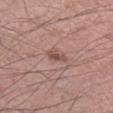Q: Is there a histopathology result?
A: imaged on a skin check; not biopsied
Q: What is the lesion's diameter?
A: about 3 mm
Q: Patient demographics?
A: male, roughly 40 years of age
Q: What is the anatomic site?
A: the right lower leg
Q: What kind of image is this?
A: ~15 mm crop, total-body skin-cancer survey
Q: How was the tile lit?
A: white-light
Q: Automated lesion metrics?
A: about 10 CIELAB-L* units darker than the surrounding skin and a normalized lesion–skin contrast near 7; a border-irregularity rating of about 3/10, a color-variation rating of about 1.5/10, and a peripheral color-asymmetry measure near 0